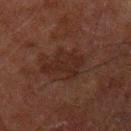Impression:
Captured during whole-body skin photography for melanoma surveillance; the lesion was not biopsied.
Context:
The lesion is on the left upper arm. Imaged with cross-polarized lighting. The subject is a male approximately 60 years of age. The lesion-visualizer software estimated an outline eccentricity of about 0.85 (0 = round, 1 = elongated) and two-axis asymmetry of about 0.5. The software also gave a mean CIELAB color near L≈18 a*≈15 b*≈18, roughly 5 lightness units darker than nearby skin, and a normalized border contrast of about 6.5. It also reported a border-irregularity rating of about 7/10, a color-variation rating of about 1.5/10, and peripheral color asymmetry of about 0.5. A close-up tile cropped from a whole-body skin photograph, about 15 mm across.